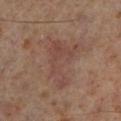No biopsy was performed on this lesion — it was imaged during a full skin examination and was not determined to be concerning. The patient is a male roughly 60 years of age. Approximately 6 mm at its widest. The lesion-visualizer software estimated a mean CIELAB color near L≈41 a*≈18 b*≈22, roughly 6 lightness units darker than nearby skin, and a normalized border contrast of about 5. A lesion tile, about 15 mm wide, cut from a 3D total-body photograph. Imaged with cross-polarized lighting. The lesion is located on the left lower leg.